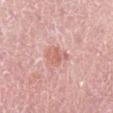Image and clinical context:
A male subject aged around 50. A roughly 15 mm field-of-view crop from a total-body skin photograph. The total-body-photography lesion software estimated a shape-asymmetry score of about 0.3 (0 = symmetric). The analysis additionally found an average lesion color of about L≈63 a*≈25 b*≈26 (CIELAB), roughly 9 lightness units darker than nearby skin, and a normalized lesion–skin contrast near 6. And it measured radial color variation of about 0.5. The recorded lesion diameter is about 3.5 mm. The lesion is on the right lower leg.